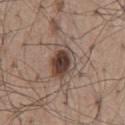The lesion was tiled from a total-body skin photograph and was not biopsied. A male patient, aged approximately 55. A 15 mm close-up tile from a total-body photography series done for melanoma screening. Captured under white-light illumination. The lesion is located on the mid back.